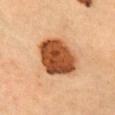Recorded during total-body skin imaging; not selected for excision or biopsy.
A roughly 15 mm field-of-view crop from a total-body skin photograph.
A female patient approximately 35 years of age.
Located on the head or neck.